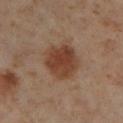This lesion was catalogued during total-body skin photography and was not selected for biopsy.
Cropped from a total-body skin-imaging series; the visible field is about 15 mm.
Automated tile analysis of the lesion measured an area of roughly 15 mm² and an outline eccentricity of about 0.3 (0 = round, 1 = elongated). The software also gave an average lesion color of about L≈42 a*≈20 b*≈29 (CIELAB), roughly 11 lightness units darker than nearby skin, and a normalized lesion–skin contrast near 9. It also reported a border-irregularity index near 1.5/10 and a within-lesion color-variation index near 3.5/10.
Approximately 4.5 mm at its widest.
From the right lower leg.
A female subject about 55 years old.
Imaged with cross-polarized lighting.